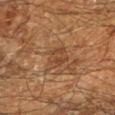Recorded during total-body skin imaging; not selected for excision or biopsy. The lesion is located on the left lower leg. Automated tile analysis of the lesion measured a mean CIELAB color near L≈33 a*≈16 b*≈27, about 6 CIELAB-L* units darker than the surrounding skin, and a lesion-to-skin contrast of about 5.5 (normalized; higher = more distinct). The analysis additionally found a border-irregularity rating of about 2/10, a color-variation rating of about 3/10, and a peripheral color-asymmetry measure near 1. The software also gave a classifier nevus-likeness of about 10/100. The tile uses cross-polarized illumination. This image is a 15 mm lesion crop taken from a total-body photograph. A male subject about 60 years old.